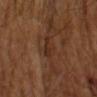biopsy status — no biopsy performed (imaged during a skin exam)
imaging modality — ~15 mm crop, total-body skin-cancer survey
diameter — ≈3 mm
lighting — cross-polarized illumination
patient — male, approximately 65 years of age